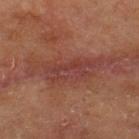The lesion was tiled from a total-body skin photograph and was not biopsied. A roughly 15 mm field-of-view crop from a total-body skin photograph. An algorithmic analysis of the crop reported a shape-asymmetry score of about 0.5 (0 = symmetric). And it measured roughly 6 lightness units darker than nearby skin and a lesion-to-skin contrast of about 6.5 (normalized; higher = more distinct). The lesion is located on the back. A male patient, in their 70s. This is a cross-polarized tile. Measured at roughly 9.5 mm in maximum diameter.